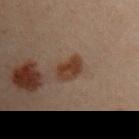Background:
On the left arm. A close-up tile cropped from a whole-body skin photograph, about 15 mm across. A female subject, approximately 30 years of age.